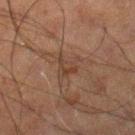Case summary:
• subject: male, about 60 years old
• image: 15 mm crop, total-body photography
• lighting: cross-polarized
• body site: the left leg
• lesion size: ~3 mm (longest diameter)